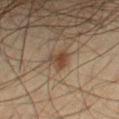  biopsy_status: not biopsied; imaged during a skin examination